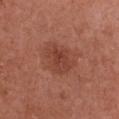<tbp_lesion>
<biopsy_status>not biopsied; imaged during a skin examination</biopsy_status>
<patient>
  <sex>female</sex>
  <age_approx>50</age_approx>
</patient>
<automated_metrics>
  <border_irregularity_0_10>2.5</border_irregularity_0_10>
  <color_variation_0_10>3.0</color_variation_0_10>
  <peripheral_color_asymmetry>1.0</peripheral_color_asymmetry>
  <nevus_likeness_0_100>15</nevus_likeness_0_100>
  <lesion_detection_confidence_0_100>100</lesion_detection_confidence_0_100>
</automated_metrics>
<image>
  <source>total-body photography crop</source>
  <field_of_view_mm>15</field_of_view_mm>
</image>
<site>chest</site>
</tbp_lesion>